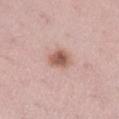Part of a total-body skin-imaging series; this lesion was reviewed on a skin check and was not flagged for biopsy.
The lesion-visualizer software estimated an average lesion color of about L≈58 a*≈22 b*≈26 (CIELAB) and a lesion-to-skin contrast of about 8.5 (normalized; higher = more distinct). The software also gave a border-irregularity index near 2/10, internal color variation of about 4 on a 0–10 scale, and a peripheral color-asymmetry measure near 1.5. The analysis additionally found a nevus-likeness score of about 95/100 and a lesion-detection confidence of about 100/100.
The lesion is on the right lower leg.
The subject is a female in their mid- to late 30s.
This image is a 15 mm lesion crop taken from a total-body photograph.
Measured at roughly 2.5 mm in maximum diameter.
Imaged with white-light lighting.